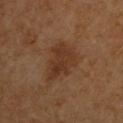Assessment: No biopsy was performed on this lesion — it was imaged during a full skin examination and was not determined to be concerning. Background: The lesion's longest dimension is about 5.5 mm. A 15 mm crop from a total-body photograph taken for skin-cancer surveillance. The subject is a male aged around 60. Located on the left upper arm. Captured under cross-polarized illumination.